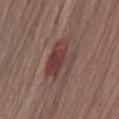Imaged during a routine full-body skin examination; the lesion was not biopsied and no histopathology is available.